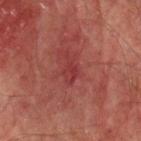The lesion was tiled from a total-body skin photograph and was not biopsied.